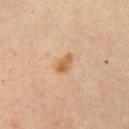No biopsy was performed on this lesion — it was imaged during a full skin examination and was not determined to be concerning. The recorded lesion diameter is about 2.5 mm. A 15 mm close-up tile from a total-body photography series done for melanoma screening. This is a cross-polarized tile. The lesion is located on the left upper arm. A male patient, in their 60s.